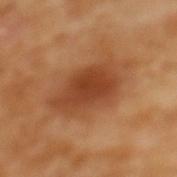biopsy_status: not biopsied; imaged during a skin examination